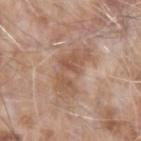Impression: The lesion was photographed on a routine skin check and not biopsied; there is no pathology result. Acquisition and patient details: A 15 mm close-up tile from a total-body photography series done for melanoma screening. About 6.5 mm across. Imaged with white-light lighting. The patient is a male aged approximately 75. From the left forearm.